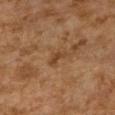Q: How large is the lesion?
A: ~2.5 mm (longest diameter)
Q: What are the patient's age and sex?
A: female, aged approximately 60
Q: Lesion location?
A: the right upper arm
Q: How was the tile lit?
A: cross-polarized
Q: What kind of image is this?
A: 15 mm crop, total-body photography